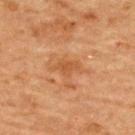Part of a total-body skin-imaging series; this lesion was reviewed on a skin check and was not flagged for biopsy.
A female subject aged 68 to 72.
The lesion is located on the back.
The tile uses cross-polarized illumination.
The recorded lesion diameter is about 2.5 mm.
Automated image analysis of the tile measured two-axis asymmetry of about 0.3. It also reported about 7 CIELAB-L* units darker than the surrounding skin and a normalized border contrast of about 5.5. It also reported a border-irregularity rating of about 3/10, a within-lesion color-variation index near 1/10, and peripheral color asymmetry of about 0.5. The software also gave a classifier nevus-likeness of about 0/100.
Cropped from a whole-body photographic skin survey; the tile spans about 15 mm.